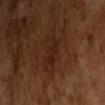Background:
The tile uses cross-polarized illumination. The lesion-visualizer software estimated an area of roughly 4 mm², a shape eccentricity near 0.8, and a shape-asymmetry score of about 0.3 (0 = symmetric). The analysis additionally found a mean CIELAB color near L≈21 a*≈20 b*≈23 and a lesion-to-skin contrast of about 6 (normalized; higher = more distinct). The software also gave a border-irregularity index near 3.5/10 and a within-lesion color-variation index near 1.5/10. The software also gave an automated nevus-likeness rating near 15 out of 100 and lesion-presence confidence of about 100/100. The subject is a male aged 63 to 67. A lesion tile, about 15 mm wide, cut from a 3D total-body photograph. About 2.5 mm across.